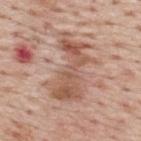notes — no biopsy performed (imaged during a skin exam)
image source — 15 mm crop, total-body photography
subject — male, aged around 75
site — the upper back